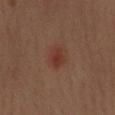No biopsy was performed on this lesion — it was imaged during a full skin examination and was not determined to be concerning. A male subject, aged 38 to 42. A roughly 15 mm field-of-view crop from a total-body skin photograph. Imaged with cross-polarized lighting. An algorithmic analysis of the crop reported an area of roughly 4.5 mm² and an outline eccentricity of about 0.65 (0 = round, 1 = elongated). Measured at roughly 2.5 mm in maximum diameter. On the left forearm.